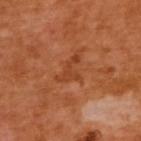| key | value |
|---|---|
| biopsy status | catalogued during a skin exam; not biopsied |
| imaging modality | ~15 mm crop, total-body skin-cancer survey |
| body site | the back |
| patient | female, in their mid- to late 50s |